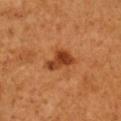This lesion was catalogued during total-body skin photography and was not selected for biopsy. A lesion tile, about 15 mm wide, cut from a 3D total-body photograph. A male patient, aged around 50. The lesion's longest dimension is about 4 mm. Located on the right upper arm. The tile uses cross-polarized illumination. Automated tile analysis of the lesion measured a lesion area of about 7.5 mm², a shape eccentricity near 0.8, and two-axis asymmetry of about 0.4. The analysis additionally found a mean CIELAB color near L≈43 a*≈29 b*≈40 and a lesion–skin lightness drop of about 12. The software also gave border irregularity of about 4 on a 0–10 scale and a color-variation rating of about 4.5/10. The analysis additionally found lesion-presence confidence of about 100/100.